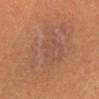Impression: Part of a total-body skin-imaging series; this lesion was reviewed on a skin check and was not flagged for biopsy. Clinical summary: A region of skin cropped from a whole-body photographic capture, roughly 15 mm wide. A female subject, aged 28–32. From the right lower leg.